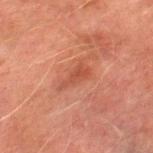Part of a total-body skin-imaging series; this lesion was reviewed on a skin check and was not flagged for biopsy.
The patient is a male roughly 75 years of age.
Approximately 3.5 mm at its widest.
The tile uses cross-polarized illumination.
From the right thigh.
A close-up tile cropped from a whole-body skin photograph, about 15 mm across.
Automated tile analysis of the lesion measured a footprint of about 4.5 mm², a shape eccentricity near 0.9, and two-axis asymmetry of about 0.45. The analysis additionally found a border-irregularity rating of about 4.5/10 and internal color variation of about 1.5 on a 0–10 scale.